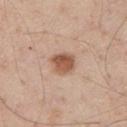Findings:
• follow-up — total-body-photography surveillance lesion; no biopsy
• illumination — white-light
• location — the chest
• subject — male, approximately 40 years of age
• size — about 3 mm
• acquisition — 15 mm crop, total-body photography
• TBP lesion metrics — a shape eccentricity near 0.5; an automated nevus-likeness rating near 100 out of 100 and lesion-presence confidence of about 100/100